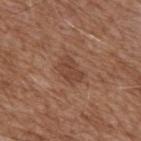Notes:
– workup: total-body-photography surveillance lesion; no biopsy
– patient: male, roughly 75 years of age
– image source: total-body-photography crop, ~15 mm field of view
– image-analysis metrics: internal color variation of about 2 on a 0–10 scale and a peripheral color-asymmetry measure near 1
– body site: the back
– tile lighting: white-light illumination